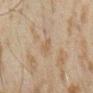<case>
  <biopsy_status>not biopsied; imaged during a skin examination</biopsy_status>
  <site>arm</site>
  <lesion_size>
    <long_diameter_mm_approx>2.5</long_diameter_mm_approx>
  </lesion_size>
  <patient>
    <sex>male</sex>
    <age_approx>45</age_approx>
  </patient>
  <image>
    <source>total-body photography crop</source>
    <field_of_view_mm>15</field_of_view_mm>
  </image>
  <automated_metrics>
    <cielab_L>47</cielab_L>
    <cielab_a>12</cielab_a>
    <cielab_b>27</cielab_b>
    <vs_skin_darker_L>5.0</vs_skin_darker_L>
  </automated_metrics>
</case>